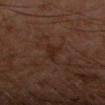The lesion was photographed on a routine skin check and not biopsied; there is no pathology result. Imaged with cross-polarized lighting. About 2.5 mm across. Cropped from a whole-body photographic skin survey; the tile spans about 15 mm. A male subject aged 58 to 62. The lesion is on the arm.